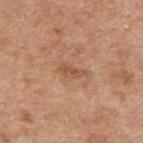biopsy status=total-body-photography surveillance lesion; no biopsy | image=15 mm crop, total-body photography | location=the right upper arm | patient=male, aged around 60.